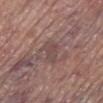| key | value |
|---|---|
| notes | no biopsy performed (imaged during a skin exam) |
| TBP lesion metrics | an area of roughly 5 mm², a shape eccentricity near 0.85, and a shape-asymmetry score of about 0.35 (0 = symmetric); border irregularity of about 4 on a 0–10 scale, internal color variation of about 3 on a 0–10 scale, and peripheral color asymmetry of about 1; a nevus-likeness score of about 0/100 and a detector confidence of about 55 out of 100 that the crop contains a lesion |
| anatomic site | the left lower leg |
| illumination | white-light illumination |
| patient | male, aged 68–72 |
| image source | total-body-photography crop, ~15 mm field of view |
| lesion diameter | ~3.5 mm (longest diameter) |